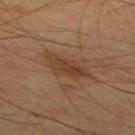notes: total-body-photography surveillance lesion; no biopsy | body site: the mid back | patient: male, about 35 years old | image: 15 mm crop, total-body photography.